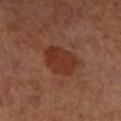Case summary:
– notes — imaged on a skin check; not biopsied
– imaging modality — ~15 mm tile from a whole-body skin photo
– automated lesion analysis — an area of roughly 13 mm², an outline eccentricity of about 0.6 (0 = round, 1 = elongated), and two-axis asymmetry of about 0.2; an average lesion color of about L≈35 a*≈25 b*≈29 (CIELAB), about 8 CIELAB-L* units darker than the surrounding skin, and a normalized border contrast of about 7.5; a border-irregularity index near 2/10 and internal color variation of about 2.5 on a 0–10 scale
– subject — female
– site — the leg
– lighting — cross-polarized
– lesion size — ~4.5 mm (longest diameter)